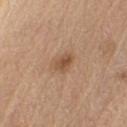{"biopsy_status": "not biopsied; imaged during a skin examination", "site": "right upper arm", "patient": {"sex": "male", "age_approx": 70}, "lighting": "white-light", "image": {"source": "total-body photography crop", "field_of_view_mm": 15}}